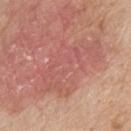This lesion was catalogued during total-body skin photography and was not selected for biopsy. The tile uses white-light illumination. A lesion tile, about 15 mm wide, cut from a 3D total-body photograph. The recorded lesion diameter is about 9 mm. The lesion is located on the back. The patient is a male aged 78 to 82. Automated tile analysis of the lesion measured an area of roughly 22 mm², an eccentricity of roughly 0.9, and a shape-asymmetry score of about 0.55 (0 = symmetric). It also reported a mean CIELAB color near L≈56 a*≈27 b*≈26, a lesion–skin lightness drop of about 6, and a lesion-to-skin contrast of about 4.5 (normalized; higher = more distinct). It also reported a border-irregularity rating of about 9/10 and a color-variation rating of about 2.5/10.